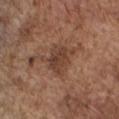The lesion was photographed on a routine skin check and not biopsied; there is no pathology result. A lesion tile, about 15 mm wide, cut from a 3D total-body photograph. The total-body-photography lesion software estimated a lesion area of about 10 mm², an outline eccentricity of about 0.5 (0 = round, 1 = elongated), and a symmetry-axis asymmetry near 0.4. The analysis additionally found a mean CIELAB color near L≈41 a*≈20 b*≈28, roughly 9 lightness units darker than nearby skin, and a lesion-to-skin contrast of about 7 (normalized; higher = more distinct). The analysis additionally found peripheral color asymmetry of about 1. It also reported an automated nevus-likeness rating near 5 out of 100 and a lesion-detection confidence of about 100/100. A male subject roughly 75 years of age. The lesion's longest dimension is about 4 mm. This is a white-light tile. The lesion is on the chest.